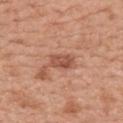Impression: This lesion was catalogued during total-body skin photography and was not selected for biopsy. Acquisition and patient details: Automated image analysis of the tile measured about 11 CIELAB-L* units darker than the surrounding skin and a normalized border contrast of about 7.5. The analysis additionally found a border-irregularity index near 2/10, a within-lesion color-variation index near 3.5/10, and a peripheral color-asymmetry measure near 1.5. A close-up tile cropped from a whole-body skin photograph, about 15 mm across. The lesion is on the back. A female patient in their mid-30s. Measured at roughly 3 mm in maximum diameter.